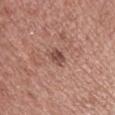workup: total-body-photography surveillance lesion; no biopsy | subject: male, roughly 30 years of age | TBP lesion metrics: roughly 11 lightness units darker than nearby skin and a lesion-to-skin contrast of about 8 (normalized; higher = more distinct); a border-irregularity rating of about 2.5/10, a within-lesion color-variation index near 2/10, and a peripheral color-asymmetry measure near 0.5; a nevus-likeness score of about 0/100 | acquisition: ~15 mm crop, total-body skin-cancer survey | size: ≈2.5 mm | lighting: white-light.